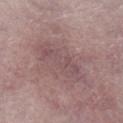The lesion was tiled from a total-body skin photograph and was not biopsied. A lesion tile, about 15 mm wide, cut from a 3D total-body photograph. Located on the left lower leg. A female subject, about 60 years old.